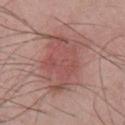Imaged during a routine full-body skin examination; the lesion was not biopsied and no histopathology is available.
From the chest.
A 15 mm close-up extracted from a 3D total-body photography capture.
A male patient in their mid- to late 50s.
The lesion's longest dimension is about 8 mm.
The tile uses white-light illumination.
An algorithmic analysis of the crop reported a lesion color around L≈52 a*≈23 b*≈23 in CIELAB, about 8 CIELAB-L* units darker than the surrounding skin, and a normalized lesion–skin contrast near 6. It also reported internal color variation of about 4.5 on a 0–10 scale and peripheral color asymmetry of about 1.5.